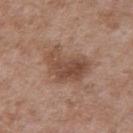The lesion was tiled from a total-body skin photograph and was not biopsied. A 15 mm crop from a total-body photograph taken for skin-cancer surveillance. From the right upper arm. Automated image analysis of the tile measured a footprint of about 15 mm², an outline eccentricity of about 0.75 (0 = round, 1 = elongated), and a shape-asymmetry score of about 0.4 (0 = symmetric). The software also gave a border-irregularity rating of about 4.5/10, a color-variation rating of about 5/10, and peripheral color asymmetry of about 2. The analysis additionally found a classifier nevus-likeness of about 35/100 and a detector confidence of about 100 out of 100 that the crop contains a lesion. Captured under white-light illumination. The patient is a male in their 50s.